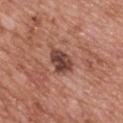Imaged during a routine full-body skin examination; the lesion was not biopsied and no histopathology is available. A 15 mm close-up extracted from a 3D total-body photography capture. The subject is a female aged approximately 60. Measured at roughly 3.5 mm in maximum diameter. The lesion is on the back.